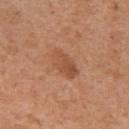Part of a total-body skin-imaging series; this lesion was reviewed on a skin check and was not flagged for biopsy. A 15 mm crop from a total-body photograph taken for skin-cancer surveillance. The lesion-visualizer software estimated border irregularity of about 2 on a 0–10 scale, a within-lesion color-variation index near 4/10, and a peripheral color-asymmetry measure near 1.5. And it measured a classifier nevus-likeness of about 35/100 and lesion-presence confidence of about 100/100. The patient is a female in their 30s. The lesion is on the right upper arm. This is a white-light tile.